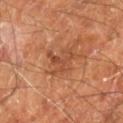Clinical impression: Part of a total-body skin-imaging series; this lesion was reviewed on a skin check and was not flagged for biopsy. Acquisition and patient details: A 15 mm close-up tile from a total-body photography series done for melanoma screening. Automated image analysis of the tile measured border irregularity of about 7 on a 0–10 scale, a color-variation rating of about 2/10, and peripheral color asymmetry of about 0.5. And it measured an automated nevus-likeness rating near 0 out of 100 and lesion-presence confidence of about 100/100. A male patient, aged 58–62. Located on the right leg.